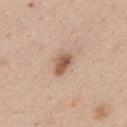Assessment:
The lesion was tiled from a total-body skin photograph and was not biopsied.
Background:
The recorded lesion diameter is about 3 mm. The lesion is on the chest. A roughly 15 mm field-of-view crop from a total-body skin photograph. A female subject aged 38–42. Automated tile analysis of the lesion measured border irregularity of about 2.5 on a 0–10 scale, a color-variation rating of about 3.5/10, and radial color variation of about 1.